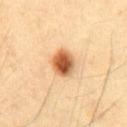Clinical impression:
This lesion was catalogued during total-body skin photography and was not selected for biopsy.
Background:
The recorded lesion diameter is about 3.5 mm. The patient is a male aged around 40. The lesion is on the chest. This is a cross-polarized tile. This image is a 15 mm lesion crop taken from a total-body photograph.